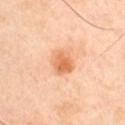Notes:
- biopsy status · imaged on a skin check; not biopsied
- patient · male, aged 43 to 47
- lesion diameter · ~3.5 mm (longest diameter)
- imaging modality · total-body-photography crop, ~15 mm field of view
- anatomic site · the left upper arm